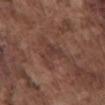This lesion was catalogued during total-body skin photography and was not selected for biopsy.
A male patient aged approximately 75.
A lesion tile, about 15 mm wide, cut from a 3D total-body photograph.
Captured under white-light illumination.
From the front of the torso.